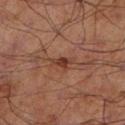  biopsy_status: not biopsied; imaged during a skin examination
  image:
    source: total-body photography crop
    field_of_view_mm: 15
  lighting: cross-polarized
  automated_metrics:
    border_irregularity_0_10: 4.0
  lesion_size:
    long_diameter_mm_approx: 2.5
  patient:
    sex: male
    age_approx: 70
  site: left lower leg